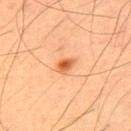Q: Is there a histopathology result?
A: catalogued during a skin exam; not biopsied
Q: What is the lesion's diameter?
A: ≈2.5 mm
Q: What lighting was used for the tile?
A: cross-polarized illumination
Q: Where on the body is the lesion?
A: the upper back
Q: What are the patient's age and sex?
A: male, about 55 years old
Q: How was this image acquired?
A: 15 mm crop, total-body photography